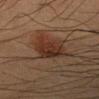Q: Is there a histopathology result?
A: imaged on a skin check; not biopsied
Q: Automated lesion metrics?
A: a border-irregularity index near 2.5/10 and internal color variation of about 4 on a 0–10 scale
Q: How was the tile lit?
A: cross-polarized
Q: What is the anatomic site?
A: the left forearm
Q: What is the imaging modality?
A: ~15 mm tile from a whole-body skin photo
Q: Patient demographics?
A: male, aged approximately 35